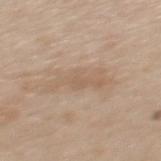Captured during whole-body skin photography for melanoma surveillance; the lesion was not biopsied. On the mid back. The patient is a male roughly 60 years of age. Cropped from a total-body skin-imaging series; the visible field is about 15 mm.